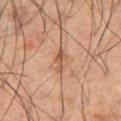Context:
Measured at roughly 3 mm in maximum diameter. A male subject roughly 60 years of age. A 15 mm close-up tile from a total-body photography series done for melanoma screening. This is a cross-polarized tile. The lesion is on the right thigh.